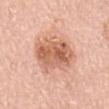{
  "biopsy_status": "not biopsied; imaged during a skin examination",
  "patient": {
    "sex": "male",
    "age_approx": 80
  },
  "lesion_size": {
    "long_diameter_mm_approx": 6.0
  },
  "image": {
    "source": "total-body photography crop",
    "field_of_view_mm": 15
  },
  "lighting": "white-light",
  "site": "mid back"
}